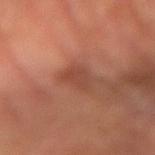biopsy status: imaged on a skin check; not biopsied | illumination: cross-polarized illumination | location: the left forearm | acquisition: ~15 mm crop, total-body skin-cancer survey | patient: male, approximately 65 years of age | lesion size: about 2.5 mm.